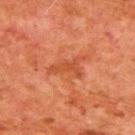Notes:
- workup: no biopsy performed (imaged during a skin exam)
- subject: male, aged around 80
- TBP lesion metrics: an area of roughly 5.5 mm² and an eccentricity of roughly 0.9; a within-lesion color-variation index near 1.5/10
- anatomic site: the upper back
- image source: ~15 mm crop, total-body skin-cancer survey
- tile lighting: cross-polarized illumination